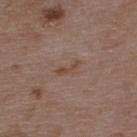Impression: Recorded during total-body skin imaging; not selected for excision or biopsy. Acquisition and patient details: The tile uses white-light illumination. A close-up tile cropped from a whole-body skin photograph, about 15 mm across. About 3 mm across. A male subject, approximately 50 years of age. The lesion is located on the upper back. Automated tile analysis of the lesion measured an area of roughly 3 mm² and an eccentricity of roughly 0.9. The software also gave a classifier nevus-likeness of about 0/100 and a lesion-detection confidence of about 100/100.